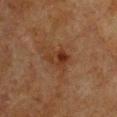  biopsy_status: not biopsied; imaged during a skin examination
  site: chest
  automated_metrics:
    border_irregularity_0_10: 3.0
    color_variation_0_10: 7.0
    peripheral_color_asymmetry: 2.5
  patient:
    sex: female
    age_approx: 55
  image:
    source: total-body photography crop
    field_of_view_mm: 15
  lighting: cross-polarized